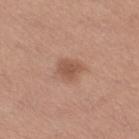Clinical impression: No biopsy was performed on this lesion — it was imaged during a full skin examination and was not determined to be concerning. Context: The lesion is located on the right thigh. Automated tile analysis of the lesion measured a mean CIELAB color near L≈53 a*≈22 b*≈29, about 10 CIELAB-L* units darker than the surrounding skin, and a normalized lesion–skin contrast near 7. A 15 mm close-up tile from a total-body photography series done for melanoma screening. Longest diameter approximately 2.5 mm. A female patient approximately 55 years of age.